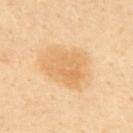Notes:
* biopsy status — total-body-photography surveillance lesion; no biopsy
* acquisition — ~15 mm tile from a whole-body skin photo
* lesion diameter — ≈6 mm
* site — the upper back
* subject — male, about 40 years old